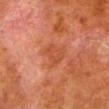biopsy status = imaged on a skin check; not biopsied
acquisition = 15 mm crop, total-body photography
patient = male, aged 78 to 82
body site = the left lower leg
lesion diameter = about 3.5 mm
tile lighting = cross-polarized